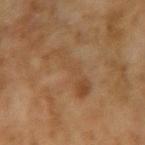Part of a total-body skin-imaging series; this lesion was reviewed on a skin check and was not flagged for biopsy. A female subject aged around 60. This is a cross-polarized tile. On the arm. A 15 mm close-up tile from a total-body photography series done for melanoma screening.